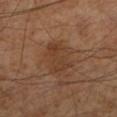No biopsy was performed on this lesion — it was imaged during a full skin examination and was not determined to be concerning.
Automated image analysis of the tile measured an area of roughly 9.5 mm² and a shape eccentricity near 0.8.
The subject is a male aged around 65.
Measured at roughly 4.5 mm in maximum diameter.
A 15 mm close-up extracted from a 3D total-body photography capture.
This is a cross-polarized tile.